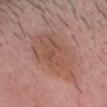Clinical impression: The lesion was tiled from a total-body skin photograph and was not biopsied. Acquisition and patient details: This image is a 15 mm lesion crop taken from a total-body photograph. Located on the head or neck. An algorithmic analysis of the crop reported a shape eccentricity near 0.8 and a symmetry-axis asymmetry near 0.25. It also reported border irregularity of about 4 on a 0–10 scale, internal color variation of about 4 on a 0–10 scale, and radial color variation of about 1.5. And it measured a classifier nevus-likeness of about 15/100 and a lesion-detection confidence of about 100/100. The patient is a male roughly 25 years of age. About 8.5 mm across.